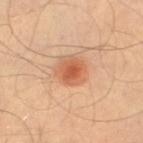Imaged during a routine full-body skin examination; the lesion was not biopsied and no histopathology is available. Cropped from a whole-body photographic skin survey; the tile spans about 15 mm. Imaged with cross-polarized lighting. The lesion is on the left thigh. A male subject, aged approximately 40. Measured at roughly 3 mm in maximum diameter.